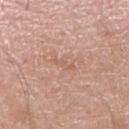Case summary:
• workup — no biopsy performed (imaged during a skin exam)
• patient — male, about 60 years old
• automated lesion analysis — a border-irregularity rating of about 5/10, internal color variation of about 0 on a 0–10 scale, and peripheral color asymmetry of about 0; a nevus-likeness score of about 0/100
• lesion size — ~3 mm (longest diameter)
• acquisition — ~15 mm crop, total-body skin-cancer survey
• tile lighting — white-light illumination
• anatomic site — the arm